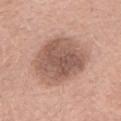A 15 mm crop from a total-body photograph taken for skin-cancer surveillance. This is a white-light tile. Measured at roughly 7 mm in maximum diameter. A female patient, approximately 60 years of age. From the head or neck. Automated tile analysis of the lesion measured lesion-presence confidence of about 100/100.